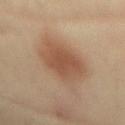{"site": "mid back", "image": {"source": "total-body photography crop", "field_of_view_mm": 15}, "patient": {"sex": "female", "age_approx": 40}, "lesion_size": {"long_diameter_mm_approx": 8.0}}